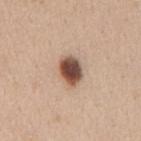- follow-up · catalogued during a skin exam; not biopsied
- acquisition · ~15 mm crop, total-body skin-cancer survey
- automated lesion analysis · a nevus-likeness score of about 100/100 and a lesion-detection confidence of about 100/100
- anatomic site · the mid back
- subject · female, aged approximately 40